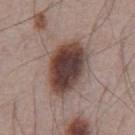* follow-up: catalogued during a skin exam; not biopsied
* size: ≈6.5 mm
* subject: male, in their mid-60s
* image: ~15 mm crop, total-body skin-cancer survey
* anatomic site: the chest
* lighting: white-light